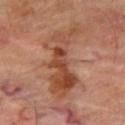Imaged during a routine full-body skin examination; the lesion was not biopsied and no histopathology is available.
A 15 mm crop from a total-body photograph taken for skin-cancer surveillance.
A male patient about 70 years old.
Located on the right thigh.